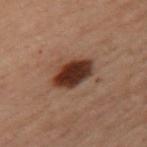notes = imaged on a skin check; not biopsied
imaging modality = 15 mm crop, total-body photography
patient = female, in their mid- to late 50s
lighting = cross-polarized illumination
body site = the left upper arm
size = ~4.5 mm (longest diameter)